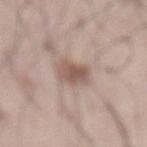Impression:
This lesion was catalogued during total-body skin photography and was not selected for biopsy.
Clinical summary:
On the abdomen. Imaged with white-light lighting. About 3.5 mm across. A male patient, approximately 55 years of age. Cropped from a whole-body photographic skin survey; the tile spans about 15 mm.